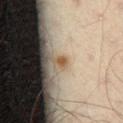The lesion was tiled from a total-body skin photograph and was not biopsied. The patient is a male about 55 years old. A region of skin cropped from a whole-body photographic capture, roughly 15 mm wide. The lesion is on the left thigh.